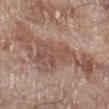Case summary:
* follow-up — total-body-photography surveillance lesion; no biopsy
* image — ~15 mm tile from a whole-body skin photo
* anatomic site — the right lower leg
* automated metrics — a footprint of about 36 mm² and two-axis asymmetry of about 0.6; a border-irregularity rating of about 9.5/10, a within-lesion color-variation index near 5.5/10, and peripheral color asymmetry of about 2
* tile lighting — white-light
* patient — male, aged 68 to 72
* size — about 11.5 mm